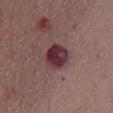<case>
<site>chest</site>
<image>
  <source>total-body photography crop</source>
  <field_of_view_mm>15</field_of_view_mm>
</image>
<patient>
  <sex>male</sex>
  <age_approx>70</age_approx>
</patient>
<lesion_size>
  <long_diameter_mm_approx>4.0</long_diameter_mm_approx>
</lesion_size>
<lighting>white-light</lighting>
<automated_metrics>
  <area_mm2_approx>9.0</area_mm2_approx>
  <eccentricity>0.6</eccentricity>
  <shape_asymmetry>0.15</shape_asymmetry>
  <nevus_likeness_0_100>10</nevus_likeness_0_100>
  <lesion_detection_confidence_0_100>100</lesion_detection_confidence_0_100>
</automated_metrics>
</case>